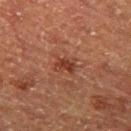Image and clinical context:
A male subject, aged around 75. Captured under cross-polarized illumination. The lesion is on the left thigh. Cropped from a whole-body photographic skin survey; the tile spans about 15 mm. Longest diameter approximately 2.5 mm. An algorithmic analysis of the crop reported a mean CIELAB color near L≈30 a*≈22 b*≈26 and a normalized border contrast of about 8. The software also gave a border-irregularity rating of about 4/10.